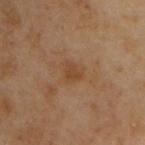Notes:
– workup: catalogued during a skin exam; not biopsied
– imaging modality: total-body-photography crop, ~15 mm field of view
– diameter: ≈2.5 mm
– automated metrics: a lesion area of about 4 mm², an eccentricity of roughly 0.65, and a symmetry-axis asymmetry near 0.35; border irregularity of about 3 on a 0–10 scale; a nevus-likeness score of about 0/100 and lesion-presence confidence of about 100/100
– body site: the chest
– subject: male, aged around 55